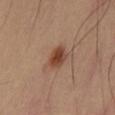| feature | finding |
|---|---|
| lesion size | ~3 mm (longest diameter) |
| image | 15 mm crop, total-body photography |
| body site | the left thigh |
| automated lesion analysis | an area of roughly 5.5 mm² and a shape eccentricity near 0.6; a lesion color around L≈44 a*≈23 b*≈30 in CIELAB, roughly 12 lightness units darker than nearby skin, and a normalized lesion–skin contrast near 9.5; a border-irregularity rating of about 1.5/10 and a color-variation rating of about 3.5/10 |
| tile lighting | cross-polarized illumination |